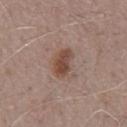  biopsy_status: not biopsied; imaged during a skin examination
  image:
    source: total-body photography crop
    field_of_view_mm: 15
  patient:
    sex: male
    age_approx: 75
  site: chest